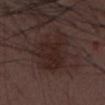The total-body-photography lesion software estimated roughly 6 lightness units darker than nearby skin and a normalized border contrast of about 7. It also reported a classifier nevus-likeness of about 50/100.
Approximately 6 mm at its widest.
The patient is a male about 30 years old.
Located on the chest.
Captured under white-light illumination.
A 15 mm close-up extracted from a 3D total-body photography capture.